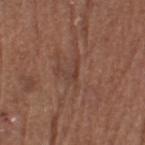Assessment: Imaged during a routine full-body skin examination; the lesion was not biopsied and no histopathology is available. Image and clinical context: A male subject aged 63 to 67. A region of skin cropped from a whole-body photographic capture, roughly 15 mm wide. The total-body-photography lesion software estimated an eccentricity of roughly 0.85 and two-axis asymmetry of about 0.65. And it measured an average lesion color of about L≈40 a*≈20 b*≈25 (CIELAB) and a normalized lesion–skin contrast near 5.5. And it measured border irregularity of about 7 on a 0–10 scale, a color-variation rating of about 0/10, and radial color variation of about 0. Located on the head or neck. This is a white-light tile. Measured at roughly 2.5 mm in maximum diameter.